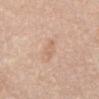Q: What are the patient's age and sex?
A: female, aged around 65
Q: Where on the body is the lesion?
A: the abdomen
Q: Lesion size?
A: ~2.5 mm (longest diameter)
Q: What did automated image analysis measure?
A: an area of roughly 3 mm², an eccentricity of roughly 0.85, and two-axis asymmetry of about 0.3; a lesion color around L≈65 a*≈19 b*≈32 in CIELAB, about 6 CIELAB-L* units darker than the surrounding skin, and a lesion-to-skin contrast of about 4.5 (normalized; higher = more distinct); a border-irregularity rating of about 3.5/10 and a peripheral color-asymmetry measure near 0.5
Q: What is the imaging modality?
A: 15 mm crop, total-body photography